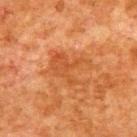{"biopsy_status": "not biopsied; imaged during a skin examination", "image": {"source": "total-body photography crop", "field_of_view_mm": 15}, "lesion_size": {"long_diameter_mm_approx": 5.5}, "site": "upper back", "patient": {"sex": "male", "age_approx": 80}}